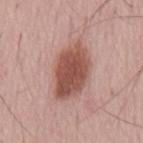The tile uses white-light illumination. A 15 mm close-up tile from a total-body photography series done for melanoma screening. The patient is a male aged approximately 55. About 7 mm across. The lesion-visualizer software estimated a lesion–skin lightness drop of about 14 and a normalized border contrast of about 10. The analysis additionally found internal color variation of about 4.5 on a 0–10 scale and radial color variation of about 1.5. The software also gave an automated nevus-likeness rating near 100 out of 100.